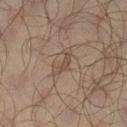Clinical impression: Recorded during total-body skin imaging; not selected for excision or biopsy. Acquisition and patient details: Located on the leg. A male subject in their mid- to late 60s. A roughly 15 mm field-of-view crop from a total-body skin photograph. Approximately 4 mm at its widest.